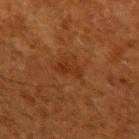biopsy status — catalogued during a skin exam; not biopsied | lesion size — about 3 mm | lighting — cross-polarized | image — total-body-photography crop, ~15 mm field of view | body site — the right upper arm | subject — male, in their 60s.